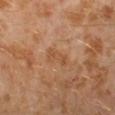follow-up: catalogued during a skin exam; not biopsied | subject: male, roughly 30 years of age | imaging modality: total-body-photography crop, ~15 mm field of view | anatomic site: the left lower leg | automated lesion analysis: a footprint of about 4 mm², a shape eccentricity near 0.9, and a symmetry-axis asymmetry near 0.25 | lighting: cross-polarized.